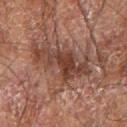Findings:
– notes: no biopsy performed (imaged during a skin exam)
– image-analysis metrics: a symmetry-axis asymmetry near 0.5; border irregularity of about 8.5 on a 0–10 scale and a within-lesion color-variation index near 3.5/10; a classifier nevus-likeness of about 20/100
– size: about 8 mm
– image source: total-body-photography crop, ~15 mm field of view
– body site: the right forearm
– illumination: cross-polarized illumination
– subject: male, aged 68–72